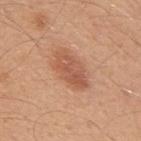Part of a total-body skin-imaging series; this lesion was reviewed on a skin check and was not flagged for biopsy.
The lesion is on the mid back.
The subject is a male aged around 50.
Automated image analysis of the tile measured a lesion area of about 10 mm², a shape eccentricity near 0.85, and a symmetry-axis asymmetry near 0.2. The analysis additionally found a lesion color around L≈56 a*≈25 b*≈32 in CIELAB, roughly 10 lightness units darker than nearby skin, and a normalized lesion–skin contrast near 6.5. It also reported an automated nevus-likeness rating near 95 out of 100 and a lesion-detection confidence of about 100/100.
The tile uses white-light illumination.
A 15 mm close-up tile from a total-body photography series done for melanoma screening.
Longest diameter approximately 5 mm.